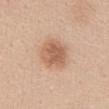{
  "biopsy_status": "not biopsied; imaged during a skin examination",
  "lighting": "white-light",
  "lesion_size": {
    "long_diameter_mm_approx": 4.0
  },
  "patient": {
    "sex": "female",
    "age_approx": 45
  },
  "site": "abdomen",
  "image": {
    "source": "total-body photography crop",
    "field_of_view_mm": 15
  }
}